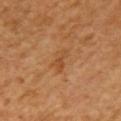Measured at roughly 3.5 mm in maximum diameter. From the right upper arm. This image is a 15 mm lesion crop taken from a total-body photograph. The patient is a female aged 53–57. Captured under cross-polarized illumination.